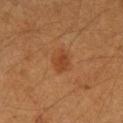Clinical impression:
Recorded during total-body skin imaging; not selected for excision or biopsy.
Context:
This is a cross-polarized tile. A female subject aged 38 to 42. On the left forearm. A region of skin cropped from a whole-body photographic capture, roughly 15 mm wide. The total-body-photography lesion software estimated a lesion color around L≈37 a*≈22 b*≈32 in CIELAB and a normalized border contrast of about 6.5.